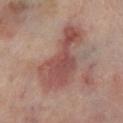Notes:
• biopsy status · catalogued during a skin exam; not biopsied
• size · about 8 mm
• body site · the left lower leg
• TBP lesion metrics · a footprint of about 23 mm², a shape eccentricity near 0.9, and a symmetry-axis asymmetry near 0.5; a border-irregularity rating of about 6.5/10, a color-variation rating of about 4.5/10, and peripheral color asymmetry of about 1.5
• image · ~15 mm crop, total-body skin-cancer survey
• illumination · cross-polarized illumination
• patient · male, aged 68–72